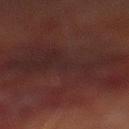Notes:
* workup — total-body-photography surveillance lesion; no biopsy
* size — ~12 mm (longest diameter)
* location — the right lower leg
* image — 15 mm crop, total-body photography
* TBP lesion metrics — a lesion–skin lightness drop of about 4 and a lesion-to-skin contrast of about 6 (normalized; higher = more distinct); a border-irregularity index near 7.5/10, a within-lesion color-variation index near 3.5/10, and peripheral color asymmetry of about 1
* patient — male, aged 68–72